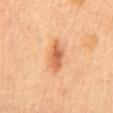  biopsy_status: not biopsied; imaged during a skin examination
  image:
    source: total-body photography crop
    field_of_view_mm: 15
  patient:
    sex: female
    age_approx: 60
  site: mid back
  automated_metrics:
    eccentricity: 0.85
    shape_asymmetry: 0.25
    border_irregularity_0_10: 2.5
    peripheral_color_asymmetry: 1.0